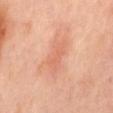Clinical impression:
Imaged during a routine full-body skin examination; the lesion was not biopsied and no histopathology is available.
Background:
A region of skin cropped from a whole-body photographic capture, roughly 15 mm wide. The lesion is on the mid back. A female subject about 50 years old. The lesion-visualizer software estimated an area of roughly 8 mm², an outline eccentricity of about 0.9 (0 = round, 1 = elongated), and a shape-asymmetry score of about 0.3 (0 = symmetric). And it measured a lesion color around L≈63 a*≈26 b*≈33 in CIELAB and a normalized lesion–skin contrast near 4.5. The software also gave a border-irregularity rating of about 4/10, internal color variation of about 2 on a 0–10 scale, and radial color variation of about 0.5.